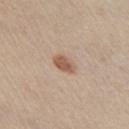Imaged during a routine full-body skin examination; the lesion was not biopsied and no histopathology is available.
The subject is a male aged approximately 55.
On the chest.
A lesion tile, about 15 mm wide, cut from a 3D total-body photograph.